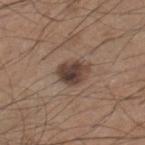  biopsy_status: not biopsied; imaged during a skin examination
  site: right lower leg
  lighting: white-light
  image:
    source: total-body photography crop
    field_of_view_mm: 15
  automated_metrics:
    area_mm2_approx: 7.5
    eccentricity: 0.55
    shape_asymmetry: 0.25
  patient:
    sex: male
    age_approx: 45